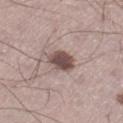The lesion was tiled from a total-body skin photograph and was not biopsied. Located on the left thigh. A male subject, roughly 45 years of age. This is a white-light tile. A 15 mm close-up extracted from a 3D total-body photography capture.